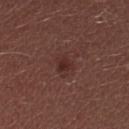Captured during whole-body skin photography for melanoma surveillance; the lesion was not biopsied. A female subject about 25 years old. The lesion's longest dimension is about 2.5 mm. Cropped from a total-body skin-imaging series; the visible field is about 15 mm. Captured under white-light illumination. The lesion is on the head or neck.